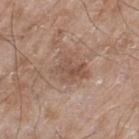Captured during whole-body skin photography for melanoma surveillance; the lesion was not biopsied.
Approximately 4.5 mm at its widest.
A lesion tile, about 15 mm wide, cut from a 3D total-body photograph.
The tile uses white-light illumination.
The total-body-photography lesion software estimated a mean CIELAB color near L≈52 a*≈18 b*≈26 and roughly 8 lightness units darker than nearby skin. And it measured an automated nevus-likeness rating near 0 out of 100.
From the left thigh.
The patient is a male in their 60s.